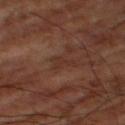Part of a total-body skin-imaging series; this lesion was reviewed on a skin check and was not flagged for biopsy.
Automated image analysis of the tile measured a border-irregularity rating of about 7/10, a color-variation rating of about 0/10, and radial color variation of about 0. And it measured a nevus-likeness score of about 0/100.
Measured at roughly 3 mm in maximum diameter.
A 15 mm close-up tile from a total-body photography series done for melanoma screening.
A subject aged around 65.
Located on the right thigh.